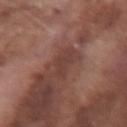Part of a total-body skin-imaging series; this lesion was reviewed on a skin check and was not flagged for biopsy. A male subject aged 73–77. A region of skin cropped from a whole-body photographic capture, roughly 15 mm wide. The lesion is located on the right forearm.